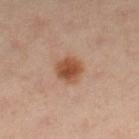biopsy status: catalogued during a skin exam; not biopsied
patient: female, roughly 40 years of age
illumination: cross-polarized
image: 15 mm crop, total-body photography
location: the leg
lesion diameter: ≈3 mm